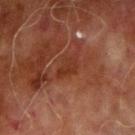* follow-up: no biopsy performed (imaged during a skin exam)
* patient: male, aged approximately 70
* anatomic site: the arm
* lighting: cross-polarized
* image: 15 mm crop, total-body photography
* lesion size: about 3 mm